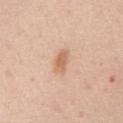No biopsy was performed on this lesion — it was imaged during a full skin examination and was not determined to be concerning. From the mid back. A male subject, aged approximately 60. A roughly 15 mm field-of-view crop from a total-body skin photograph.